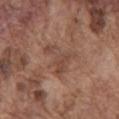Captured under white-light illumination. The lesion is on the mid back. About 4.5 mm across. The patient is a male aged around 75. A 15 mm crop from a total-body photograph taken for skin-cancer surveillance.